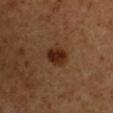  biopsy_status: not biopsied; imaged during a skin examination
  automated_metrics:
    area_mm2_approx: 6.5
    eccentricity: 0.65
    shape_asymmetry: 0.1
    cielab_L: 27
    cielab_a: 20
    cielab_b: 29
    vs_skin_darker_L: 10.0
    vs_skin_contrast_norm: 10.5
    nevus_likeness_0_100: 100
    lesion_detection_confidence_0_100: 100
  patient:
    sex: male
    age_approx: 50
  site: chest
  lighting: cross-polarized
  lesion_size:
    long_diameter_mm_approx: 3.5
  image:
    source: total-body photography crop
    field_of_view_mm: 15